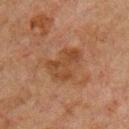| field | value |
|---|---|
| biopsy status | catalogued during a skin exam; not biopsied |
| lesion size | about 4.5 mm |
| image | 15 mm crop, total-body photography |
| site | the chest |
| subject | male, approximately 60 years of age |
| lighting | cross-polarized |